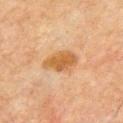- workup: total-body-photography surveillance lesion; no biopsy
- lighting: cross-polarized
- site: the chest
- subject: male, aged around 65
- size: ~4 mm (longest diameter)
- imaging modality: 15 mm crop, total-body photography